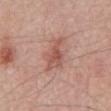Recorded during total-body skin imaging; not selected for excision or biopsy. The lesion's longest dimension is about 5.5 mm. The lesion-visualizer software estimated a within-lesion color-variation index near 3.5/10. And it measured a lesion-detection confidence of about 100/100. A male subject, aged 68 to 72. The lesion is on the mid back. This image is a 15 mm lesion crop taken from a total-body photograph.